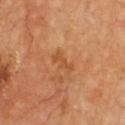biopsy status: imaged on a skin check; not biopsied
size: about 3 mm
location: the chest
TBP lesion metrics: an area of roughly 3.5 mm², an outline eccentricity of about 0.9 (0 = round, 1 = elongated), and a symmetry-axis asymmetry near 0.45; an average lesion color of about L≈53 a*≈27 b*≈42 (CIELAB) and a lesion-to-skin contrast of about 5.5 (normalized; higher = more distinct); a border-irregularity index near 6/10, a within-lesion color-variation index near 0/10, and a peripheral color-asymmetry measure near 0
imaging modality: total-body-photography crop, ~15 mm field of view